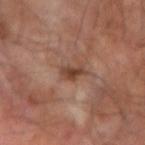This image is a 15 mm lesion crop taken from a total-body photograph.
A male subject aged 63 to 67.
On the right forearm.
The tile uses cross-polarized illumination.